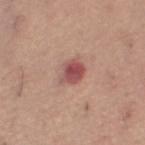{
  "biopsy_status": "not biopsied; imaged during a skin examination",
  "patient": {
    "sex": "female",
    "age_approx": 65
  },
  "lighting": "white-light",
  "image": {
    "source": "total-body photography crop",
    "field_of_view_mm": 15
  },
  "site": "left thigh"
}